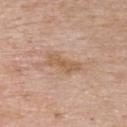The lesion was tiled from a total-body skin photograph and was not biopsied. Longest diameter approximately 4.5 mm. An algorithmic analysis of the crop reported a lesion area of about 6.5 mm², a shape eccentricity near 0.95, and two-axis asymmetry of about 0.4. The software also gave border irregularity of about 5 on a 0–10 scale and peripheral color asymmetry of about 0.5. Cropped from a total-body skin-imaging series; the visible field is about 15 mm. On the chest. This is a white-light tile. A male patient aged around 60.